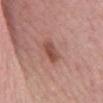This image is a 15 mm lesion crop taken from a total-body photograph. The tile uses white-light illumination. From the mid back. A female subject about 60 years old. The recorded lesion diameter is about 4.5 mm. The total-body-photography lesion software estimated a lesion area of about 5.5 mm², an outline eccentricity of about 0.95 (0 = round, 1 = elongated), and a symmetry-axis asymmetry near 0.3. The analysis additionally found a nevus-likeness score of about 45/100.